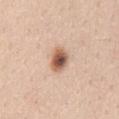Q: Was a biopsy performed?
A: no biopsy performed (imaged during a skin exam)
Q: What is the imaging modality?
A: ~15 mm crop, total-body skin-cancer survey
Q: What are the patient's age and sex?
A: female, roughly 40 years of age
Q: How large is the lesion?
A: about 3.5 mm
Q: How was the tile lit?
A: white-light
Q: Lesion location?
A: the mid back
Q: What did automated image analysis measure?
A: a lesion color around L≈59 a*≈21 b*≈30 in CIELAB, about 17 CIELAB-L* units darker than the surrounding skin, and a normalized border contrast of about 10.5; border irregularity of about 2 on a 0–10 scale, internal color variation of about 8 on a 0–10 scale, and radial color variation of about 2; a classifier nevus-likeness of about 100/100 and a lesion-detection confidence of about 100/100